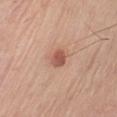Imaged during a routine full-body skin examination; the lesion was not biopsied and no histopathology is available.
A region of skin cropped from a whole-body photographic capture, roughly 15 mm wide.
Located on the left upper arm.
Longest diameter approximately 2.5 mm.
The tile uses white-light illumination.
The patient is a male aged 58–62.
An algorithmic analysis of the crop reported an area of roughly 4 mm², an outline eccentricity of about 0.7 (0 = round, 1 = elongated), and a symmetry-axis asymmetry near 0.15. The analysis additionally found an average lesion color of about L≈55 a*≈25 b*≈29 (CIELAB), a lesion–skin lightness drop of about 12, and a lesion-to-skin contrast of about 8 (normalized; higher = more distinct). The software also gave an automated nevus-likeness rating near 90 out of 100 and a detector confidence of about 100 out of 100 that the crop contains a lesion.